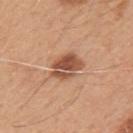Part of a total-body skin-imaging series; this lesion was reviewed on a skin check and was not flagged for biopsy.
A 15 mm close-up tile from a total-body photography series done for melanoma screening.
Captured under white-light illumination.
On the mid back.
Automated image analysis of the tile measured a lesion color around L≈52 a*≈24 b*≈32 in CIELAB, a lesion–skin lightness drop of about 14, and a normalized lesion–skin contrast near 9.5. And it measured a border-irregularity rating of about 2/10 and a within-lesion color-variation index near 5/10.
Approximately 4 mm at its widest.
A male subject aged around 50.